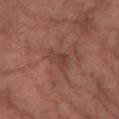- notes — catalogued during a skin exam; not biopsied
- illumination — cross-polarized
- subject — female, in their 60s
- image source — ~15 mm tile from a whole-body skin photo
- body site — the left lower leg
- diameter — ≈2.5 mm